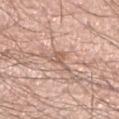Assessment:
Recorded during total-body skin imaging; not selected for excision or biopsy.
Context:
Automated tile analysis of the lesion measured an area of roughly 4 mm², a shape eccentricity near 0.85, and a symmetry-axis asymmetry near 0.55. It also reported a mean CIELAB color near L≈60 a*≈19 b*≈28, a lesion–skin lightness drop of about 10, and a normalized lesion–skin contrast near 6.5. The analysis additionally found a nevus-likeness score of about 0/100 and a lesion-detection confidence of about 75/100. A 15 mm crop from a total-body photograph taken for skin-cancer surveillance. The patient is a male about 40 years old. On the left lower leg. The tile uses white-light illumination. Measured at roughly 3.5 mm in maximum diameter.